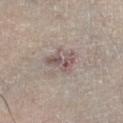This lesion was catalogued during total-body skin photography and was not selected for biopsy. A male patient, in their 70s. Located on the right lower leg. Automated tile analysis of the lesion measured a lesion color around L≈53 a*≈14 b*≈18 in CIELAB, a lesion–skin lightness drop of about 10, and a normalized lesion–skin contrast near 7.5. It also reported a within-lesion color-variation index near 4.5/10 and peripheral color asymmetry of about 1.5. The analysis additionally found a nevus-likeness score of about 0/100 and a lesion-detection confidence of about 10/100. Cropped from a whole-body photographic skin survey; the tile spans about 15 mm.